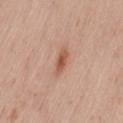Imaged during a routine full-body skin examination; the lesion was not biopsied and no histopathology is available. A female patient, aged approximately 30. Captured under white-light illumination. Located on the lower back. The lesion-visualizer software estimated an outline eccentricity of about 0.9 (0 = round, 1 = elongated). And it measured border irregularity of about 2.5 on a 0–10 scale, a within-lesion color-variation index near 2/10, and peripheral color asymmetry of about 0.5. And it measured an automated nevus-likeness rating near 90 out of 100 and a lesion-detection confidence of about 100/100. About 3 mm across. A region of skin cropped from a whole-body photographic capture, roughly 15 mm wide.